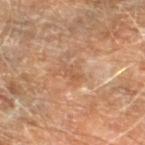Case summary:
• workup — total-body-photography surveillance lesion; no biopsy
• site — the left lower leg
• lighting — cross-polarized illumination
• patient — male, aged 68–72
• imaging modality — total-body-photography crop, ~15 mm field of view
• TBP lesion metrics — a lesion area of about 3 mm² and a shape-asymmetry score of about 0.4 (0 = symmetric); an average lesion color of about L≈55 a*≈20 b*≈34 (CIELAB), a lesion–skin lightness drop of about 7, and a lesion-to-skin contrast of about 5 (normalized; higher = more distinct)